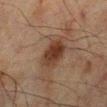  biopsy_status: not biopsied; imaged during a skin examination
  lighting: cross-polarized
  patient:
    sex: male
    age_approx: 65
  image:
    source: total-body photography crop
    field_of_view_mm: 15
  site: right lower leg